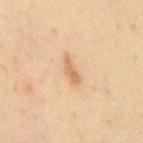Findings:
- notes — catalogued during a skin exam; not biopsied
- imaging modality — ~15 mm tile from a whole-body skin photo
- subject — male, aged approximately 50
- location — the mid back
- lesion diameter — ~3.5 mm (longest diameter)